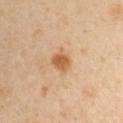Clinical impression: No biopsy was performed on this lesion — it was imaged during a full skin examination and was not determined to be concerning. Acquisition and patient details: A male patient, roughly 40 years of age. A 15 mm close-up extracted from a 3D total-body photography capture. The lesion is on the left upper arm. This is a cross-polarized tile.